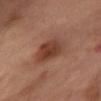The lesion was photographed on a routine skin check and not biopsied; there is no pathology result. From the abdomen. Captured under cross-polarized illumination. Automated tile analysis of the lesion measured an average lesion color of about L≈41 a*≈23 b*≈29 (CIELAB), a lesion–skin lightness drop of about 10, and a normalized lesion–skin contrast near 8.5. The software also gave a border-irregularity rating of about 2/10, a within-lesion color-variation index near 4/10, and radial color variation of about 1.5. The patient is a female in their mid- to late 50s. A roughly 15 mm field-of-view crop from a total-body skin photograph.